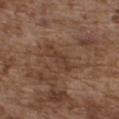biopsy status: catalogued during a skin exam; not biopsied
illumination: white-light illumination
site: the front of the torso
acquisition: ~15 mm crop, total-body skin-cancer survey
subject: male, in their mid-70s
lesion size: ≈4.5 mm